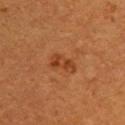workup: imaged on a skin check; not biopsied | anatomic site: the upper back | image: ~15 mm crop, total-body skin-cancer survey | subject: female, aged approximately 55.